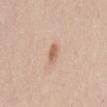Recorded during total-body skin imaging; not selected for excision or biopsy. The lesion-visualizer software estimated a footprint of about 3 mm² and an outline eccentricity of about 0.9 (0 = round, 1 = elongated). The software also gave a mean CIELAB color near L≈62 a*≈20 b*≈32, roughly 11 lightness units darker than nearby skin, and a lesion-to-skin contrast of about 7.5 (normalized; higher = more distinct). The analysis additionally found border irregularity of about 3 on a 0–10 scale, a within-lesion color-variation index near 0.5/10, and peripheral color asymmetry of about 0. It also reported a classifier nevus-likeness of about 85/100. The lesion is on the chest. Measured at roughly 3 mm in maximum diameter. The tile uses white-light illumination. The subject is a male aged around 50. A region of skin cropped from a whole-body photographic capture, roughly 15 mm wide.